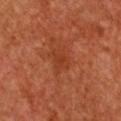follow-up = imaged on a skin check; not biopsied | location = the chest | subject = female, aged 63 to 67 | acquisition = 15 mm crop, total-body photography | illumination = cross-polarized | lesion size = ≈3 mm | automated lesion analysis = an average lesion color of about L≈39 a*≈30 b*≈37 (CIELAB), about 6 CIELAB-L* units darker than the surrounding skin, and a normalized lesion–skin contrast near 5; a border-irregularity rating of about 3/10, internal color variation of about 2 on a 0–10 scale, and radial color variation of about 0.5.